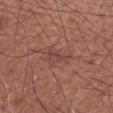acquisition = total-body-photography crop, ~15 mm field of view
subject = male, aged approximately 70
anatomic site = the right upper arm
tile lighting = white-light
diameter = ≈3 mm
automated metrics = a footprint of about 4.5 mm² and two-axis asymmetry of about 0.5; a lesion color around L≈44 a*≈23 b*≈24 in CIELAB and about 6 CIELAB-L* units darker than the surrounding skin; border irregularity of about 5 on a 0–10 scale, a color-variation rating of about 2.5/10, and peripheral color asymmetry of about 1